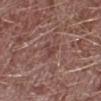Clinical impression:
Recorded during total-body skin imaging; not selected for excision or biopsy.
Background:
On the right lower leg. The patient is a male aged 43–47. About 2.5 mm across. This is a white-light tile. A 15 mm close-up tile from a total-body photography series done for melanoma screening.